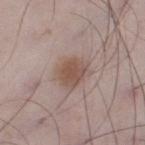Part of a total-body skin-imaging series; this lesion was reviewed on a skin check and was not flagged for biopsy.
Longest diameter approximately 4 mm.
A male patient aged 48 to 52.
The tile uses white-light illumination.
From the left lower leg.
Cropped from a whole-body photographic skin survey; the tile spans about 15 mm.